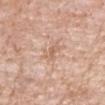biopsy status: imaged on a skin check; not biopsied
image source: ~15 mm tile from a whole-body skin photo
location: the right forearm
lesion diameter: about 3 mm
subject: female, approximately 70 years of age